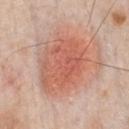Image and clinical context: A male patient, about 60 years old. This is a white-light tile. The lesion's longest dimension is about 8 mm. A 15 mm close-up tile from a total-body photography series done for melanoma screening. Automated tile analysis of the lesion measured a footprint of about 37 mm², an outline eccentricity of about 0.55 (0 = round, 1 = elongated), and a shape-asymmetry score of about 0.2 (0 = symmetric). And it measured an average lesion color of about L≈60 a*≈26 b*≈31 (CIELAB) and about 10 CIELAB-L* units darker than the surrounding skin. The analysis additionally found a border-irregularity rating of about 3/10 and internal color variation of about 4.5 on a 0–10 scale. The analysis additionally found a nevus-likeness score of about 100/100 and a detector confidence of about 100 out of 100 that the crop contains a lesion. The lesion is located on the front of the torso.